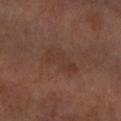Part of a total-body skin-imaging series; this lesion was reviewed on a skin check and was not flagged for biopsy. The lesion-visualizer software estimated a mean CIELAB color near L≈34 a*≈18 b*≈23, about 5 CIELAB-L* units darker than the surrounding skin, and a normalized border contrast of about 5. It also reported a border-irregularity rating of about 3.5/10, internal color variation of about 2.5 on a 0–10 scale, and a peripheral color-asymmetry measure near 1. It also reported a nevus-likeness score of about 0/100 and a lesion-detection confidence of about 100/100. The subject is a male approximately 70 years of age. The lesion is on the arm. Cropped from a whole-body photographic skin survey; the tile spans about 15 mm.